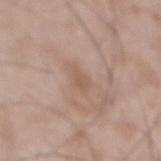Captured during whole-body skin photography for melanoma surveillance; the lesion was not biopsied. Located on the mid back. The tile uses white-light illumination. Cropped from a whole-body photographic skin survey; the tile spans about 15 mm. A male patient, aged around 50. Measured at roughly 2.5 mm in maximum diameter.